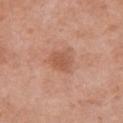Q: Was this lesion biopsied?
A: catalogued during a skin exam; not biopsied
Q: What kind of image is this?
A: 15 mm crop, total-body photography
Q: Patient demographics?
A: female, aged 48–52
Q: Lesion location?
A: the front of the torso